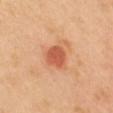The lesion was photographed on a routine skin check and not biopsied; there is no pathology result. A female patient, approximately 40 years of age. Automated tile analysis of the lesion measured a lesion area of about 7 mm², an eccentricity of roughly 0.6, and a shape-asymmetry score of about 0.2 (0 = symmetric). A 15 mm close-up extracted from a 3D total-body photography capture. The tile uses cross-polarized illumination. On the front of the torso.